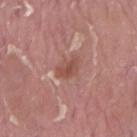The lesion was tiled from a total-body skin photograph and was not biopsied.